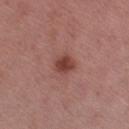Imaged during a routine full-body skin examination; the lesion was not biopsied and no histopathology is available. A male patient, about 55 years old. Imaged with white-light lighting. Measured at roughly 2.5 mm in maximum diameter. A region of skin cropped from a whole-body photographic capture, roughly 15 mm wide. An algorithmic analysis of the crop reported a lesion area of about 5 mm², an eccentricity of roughly 0.35, and a shape-asymmetry score of about 0.2 (0 = symmetric). Located on the left upper arm.